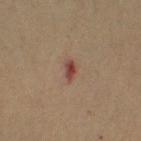Impression: Part of a total-body skin-imaging series; this lesion was reviewed on a skin check and was not flagged for biopsy. Image and clinical context: The tile uses cross-polarized illumination. The lesion-visualizer software estimated a lesion area of about 3 mm², an eccentricity of roughly 0.8, and a symmetry-axis asymmetry near 0.3. It also reported a border-irregularity rating of about 3/10, internal color variation of about 3.5 on a 0–10 scale, and peripheral color asymmetry of about 1. A lesion tile, about 15 mm wide, cut from a 3D total-body photograph. From the left arm. A male subject, aged around 55.